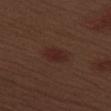Assessment:
No biopsy was performed on this lesion — it was imaged during a full skin examination and was not determined to be concerning.
Clinical summary:
The lesion-visualizer software estimated a border-irregularity index near 2/10, a within-lesion color-variation index near 2.5/10, and radial color variation of about 1. The software also gave an automated nevus-likeness rating near 90 out of 100. A 15 mm close-up extracted from a 3D total-body photography capture. Located on the left upper arm. A male patient, about 70 years old.